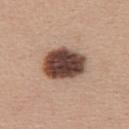This lesion was catalogued during total-body skin photography and was not selected for biopsy. From the upper back. A female subject, in their 40s. The lesion's longest dimension is about 5 mm. A roughly 15 mm field-of-view crop from a total-body skin photograph.